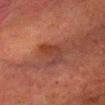This lesion was catalogued during total-body skin photography and was not selected for biopsy. The lesion-visualizer software estimated an area of roughly 6 mm², a shape eccentricity near 0.5, and two-axis asymmetry of about 0.45. The analysis additionally found an average lesion color of about L≈31 a*≈22 b*≈23 (CIELAB), roughly 6 lightness units darker than nearby skin, and a lesion-to-skin contrast of about 6 (normalized; higher = more distinct). The software also gave an automated nevus-likeness rating near 0 out of 100 and a detector confidence of about 50 out of 100 that the crop contains a lesion. About 3 mm across. The subject is a male in their 50s. On the head or neck. Cropped from a whole-body photographic skin survey; the tile spans about 15 mm.